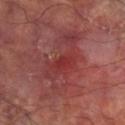Q: Was this lesion biopsied?
A: catalogued during a skin exam; not biopsied
Q: What is the imaging modality?
A: ~15 mm crop, total-body skin-cancer survey
Q: Where on the body is the lesion?
A: the leg
Q: How large is the lesion?
A: about 2.5 mm
Q: What lighting was used for the tile?
A: cross-polarized
Q: What are the patient's age and sex?
A: male, aged around 60